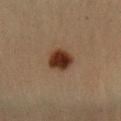Clinical impression: The lesion was photographed on a routine skin check and not biopsied; there is no pathology result. Clinical summary: A 15 mm close-up extracted from a 3D total-body photography capture. A female patient, approximately 45 years of age. From the left forearm.